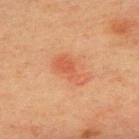This lesion was catalogued during total-body skin photography and was not selected for biopsy. On the upper back. Captured under cross-polarized illumination. A 15 mm crop from a total-body photograph taken for skin-cancer surveillance. A female subject, aged 63 to 67. Automated image analysis of the tile measured a shape eccentricity near 0.9 and two-axis asymmetry of about 0.4. The software also gave an automated nevus-likeness rating near 80 out of 100 and a lesion-detection confidence of about 100/100. Measured at roughly 4.5 mm in maximum diameter.